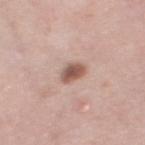Q: Was this lesion biopsied?
A: imaged on a skin check; not biopsied
Q: How large is the lesion?
A: ≈2.5 mm
Q: What kind of image is this?
A: ~15 mm crop, total-body skin-cancer survey
Q: Patient demographics?
A: female, aged 38–42
Q: What is the anatomic site?
A: the left thigh